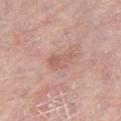workup = imaged on a skin check; not biopsied | image source = ~15 mm crop, total-body skin-cancer survey | anatomic site = the leg | subject = female, in their 70s.